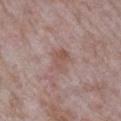<record>
<biopsy_status>not biopsied; imaged during a skin examination</biopsy_status>
<site>right upper arm</site>
<image>
  <source>total-body photography crop</source>
  <field_of_view_mm>15</field_of_view_mm>
</image>
<automated_metrics>
  <cielab_L>53</cielab_L>
  <cielab_a>19</cielab_a>
  <cielab_b>23</cielab_b>
  <vs_skin_darker_L>7.0</vs_skin_darker_L>
  <vs_skin_contrast_norm>6.0</vs_skin_contrast_norm>
  <color_variation_0_10>3.0</color_variation_0_10>
</automated_metrics>
<lesion_size>
  <long_diameter_mm_approx>3.0</long_diameter_mm_approx>
</lesion_size>
<patient>
  <sex>male</sex>
  <age_approx>65</age_approx>
</patient>
</record>